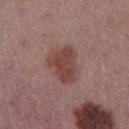Context:
Imaged with white-light lighting. Automated tile analysis of the lesion measured a shape-asymmetry score of about 0.35 (0 = symmetric). A female patient, in their mid- to late 50s. From the left thigh. Cropped from a total-body skin-imaging series; the visible field is about 15 mm. Measured at roughly 4.5 mm in maximum diameter.